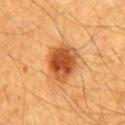{
  "biopsy_status": "not biopsied; imaged during a skin examination",
  "lesion_size": {
    "long_diameter_mm_approx": 4.5
  },
  "patient": {
    "sex": "male",
    "age_approx": 60
  },
  "lighting": "cross-polarized",
  "image": {
    "source": "total-body photography crop",
    "field_of_view_mm": 15
  },
  "site": "chest",
  "automated_metrics": {
    "border_irregularity_0_10": 2.0
  }
}